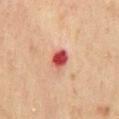Case summary:
* lesion diameter: ≈2.5 mm
* site: the mid back
* imaging modality: 15 mm crop, total-body photography
* automated lesion analysis: a lesion area of about 4.5 mm², an outline eccentricity of about 0.55 (0 = round, 1 = elongated), and a symmetry-axis asymmetry near 0.15; an average lesion color of about L≈45 a*≈33 b*≈26 (CIELAB), about 16 CIELAB-L* units darker than the surrounding skin, and a normalized lesion–skin contrast near 11.5; a classifier nevus-likeness of about 0/100 and a detector confidence of about 100 out of 100 that the crop contains a lesion
* lighting: cross-polarized
* subject: male, approximately 45 years of age